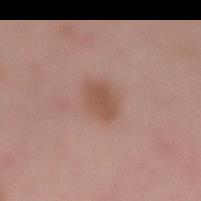This lesion was catalogued during total-body skin photography and was not selected for biopsy. A male patient, roughly 40 years of age. A lesion tile, about 15 mm wide, cut from a 3D total-body photograph. Captured under white-light illumination. The lesion is on the lower back.